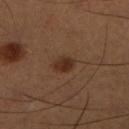No biopsy was performed on this lesion — it was imaged during a full skin examination and was not determined to be concerning.
A male subject in their 50s.
The total-body-photography lesion software estimated a lesion area of about 4 mm² and a shape eccentricity near 0.7. It also reported an average lesion color of about L≈29 a*≈19 b*≈27 (CIELAB), about 9 CIELAB-L* units darker than the surrounding skin, and a normalized border contrast of about 9. It also reported a nevus-likeness score of about 95/100.
Located on the right lower leg.
A 15 mm close-up extracted from a 3D total-body photography capture.